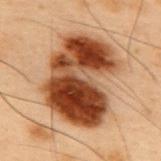Case summary:
• lighting · cross-polarized illumination
• location · the back
• size · ≈9 mm
• acquisition · 15 mm crop, total-body photography
• automated metrics · an automated nevus-likeness rating near 100 out of 100
• patient · male, aged 53 to 57